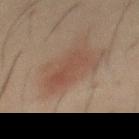follow-up=catalogued during a skin exam; not biopsied | site=the chest | subject=male, roughly 60 years of age | image=~15 mm crop, total-body skin-cancer survey.